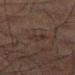notes: total-body-photography surveillance lesion; no biopsy | diameter: ≈3 mm | image: total-body-photography crop, ~15 mm field of view | subject: male, aged approximately 70 | tile lighting: cross-polarized illumination | body site: the left thigh.